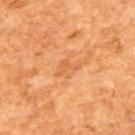Approximately 2.5 mm at its widest.
A 15 mm close-up extracted from a 3D total-body photography capture.
A male patient, aged 63 to 67.
The tile uses cross-polarized illumination.
Automated tile analysis of the lesion measured a classifier nevus-likeness of about 0/100.